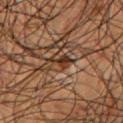Part of a total-body skin-imaging series; this lesion was reviewed on a skin check and was not flagged for biopsy.
An algorithmic analysis of the crop reported a mean CIELAB color near L≈31 a*≈19 b*≈27, a lesion–skin lightness drop of about 11, and a lesion-to-skin contrast of about 10.5 (normalized; higher = more distinct). And it measured a border-irregularity rating of about 6/10, a within-lesion color-variation index near 3/10, and a peripheral color-asymmetry measure near 1. It also reported an automated nevus-likeness rating near 5 out of 100 and a lesion-detection confidence of about 60/100.
Cropped from a whole-body photographic skin survey; the tile spans about 15 mm.
From the chest.
Longest diameter approximately 3 mm.
A male patient, in their mid- to late 50s.